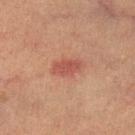The lesion was tiled from a total-body skin photograph and was not biopsied.
Approximately 3.5 mm at its widest.
A region of skin cropped from a whole-body photographic capture, roughly 15 mm wide.
From the leg.
An algorithmic analysis of the crop reported an area of roughly 5 mm², a shape eccentricity near 0.85, and a symmetry-axis asymmetry near 0.2. The software also gave an average lesion color of about L≈48 a*≈27 b*≈28 (CIELAB) and a lesion–skin lightness drop of about 9. It also reported a border-irregularity rating of about 2/10, internal color variation of about 1.5 on a 0–10 scale, and peripheral color asymmetry of about 0.5. It also reported lesion-presence confidence of about 100/100.
A female subject, aged approximately 60.